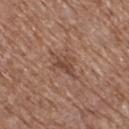| feature | finding |
|---|---|
| notes | catalogued during a skin exam; not biopsied |
| automated metrics | a lesion area of about 5.5 mm² and a shape-asymmetry score of about 0.45 (0 = symmetric); a mean CIELAB color near L≈46 a*≈20 b*≈27, a lesion–skin lightness drop of about 8, and a normalized border contrast of about 6; a border-irregularity index near 5/10, a within-lesion color-variation index near 3/10, and peripheral color asymmetry of about 1 |
| patient | female, aged 73 to 77 |
| location | the right thigh |
| lighting | white-light |
| image source | ~15 mm crop, total-body skin-cancer survey |